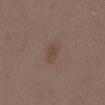<case>
  <biopsy_status>not biopsied; imaged during a skin examination</biopsy_status>
  <lighting>white-light</lighting>
  <patient>
    <sex>female</sex>
    <age_approx>35</age_approx>
  </patient>
  <automated_metrics>
    <vs_skin_contrast_norm>5.5</vs_skin_contrast_norm>
    <border_irregularity_0_10>3.0</border_irregularity_0_10>
    <color_variation_0_10>0.5</color_variation_0_10>
    <peripheral_color_asymmetry>0.0</peripheral_color_asymmetry>
    <nevus_likeness_0_100>0</nevus_likeness_0_100>
    <lesion_detection_confidence_0_100>100</lesion_detection_confidence_0_100>
  </automated_metrics>
  <site>chest</site>
  <image>
    <source>total-body photography crop</source>
    <field_of_view_mm>15</field_of_view_mm>
  </image>
</case>